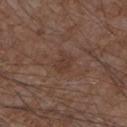<tbp_lesion>
  <biopsy_status>not biopsied; imaged during a skin examination</biopsy_status>
  <automated_metrics>
    <eccentricity>0.75</eccentricity>
    <cielab_L>36</cielab_L>
    <cielab_a>18</cielab_a>
    <cielab_b>24</cielab_b>
    <vs_skin_darker_L>5.0</vs_skin_darker_L>
    <border_irregularity_0_10>3.5</border_irregularity_0_10>
    <color_variation_0_10>2.5</color_variation_0_10>
    <peripheral_color_asymmetry>1.0</peripheral_color_asymmetry>
  </automated_metrics>
  <image>
    <source>total-body photography crop</source>
    <field_of_view_mm>15</field_of_view_mm>
  </image>
  <patient>
    <sex>male</sex>
    <age_approx>75</age_approx>
  </patient>
  <lighting>white-light</lighting>
  <lesion_size>
    <long_diameter_mm_approx>3.0</long_diameter_mm_approx>
  </lesion_size>
  <site>right forearm</site>
</tbp_lesion>